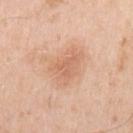  automated_metrics:
    eccentricity: 0.6
    cielab_L: 66
    cielab_a: 22
    cielab_b: 34
    vs_skin_darker_L: 8.0
    vs_skin_contrast_norm: 5.0
    color_variation_0_10: 3.0
  lighting: white-light
  patient:
    sex: male
    age_approx: 70
  lesion_size:
    long_diameter_mm_approx: 4.5
  site: left upper arm
  image:
    source: total-body photography crop
    field_of_view_mm: 15